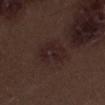site: the right thigh
acquisition: ~15 mm crop, total-body skin-cancer survey
patient: male, aged 68–72